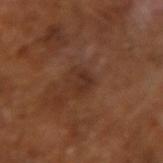Case summary:
* notes · catalogued during a skin exam; not biopsied
* acquisition · ~15 mm tile from a whole-body skin photo
* lesion size · ≈3 mm
* patient · male, about 65 years old